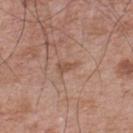biopsy status: catalogued during a skin exam; not biopsied
subject: male, in their mid- to late 60s
lesion size: about 2.5 mm
acquisition: 15 mm crop, total-body photography
body site: the upper back
tile lighting: white-light